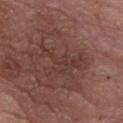Clinical impression: Imaged during a routine full-body skin examination; the lesion was not biopsied and no histopathology is available. Image and clinical context: The recorded lesion diameter is about 5.5 mm. A male subject, aged approximately 75. Cropped from a whole-body photographic skin survey; the tile spans about 15 mm. The lesion is on the chest. Automated tile analysis of the lesion measured an area of roughly 9 mm² and a shape eccentricity near 0.85. It also reported an average lesion color of about L≈36 a*≈20 b*≈21 (CIELAB) and roughly 5 lightness units darker than nearby skin. The analysis additionally found lesion-presence confidence of about 95/100. Captured under white-light illumination.